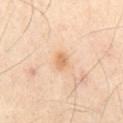A male subject, aged 53 to 57. Longest diameter approximately 2.5 mm. From the chest. A 15 mm close-up tile from a total-body photography series done for melanoma screening.